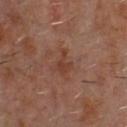<tbp_lesion>
<biopsy_status>not biopsied; imaged during a skin examination</biopsy_status>
<site>front of the torso</site>
<patient>
  <sex>male</sex>
  <age_approx>60</age_approx>
</patient>
<lesion_size>
  <long_diameter_mm_approx>3.0</long_diameter_mm_approx>
</lesion_size>
<lighting>cross-polarized</lighting>
<image>
  <source>total-body photography crop</source>
  <field_of_view_mm>15</field_of_view_mm>
</image>
</tbp_lesion>